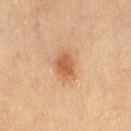Q: Is there a histopathology result?
A: total-body-photography surveillance lesion; no biopsy